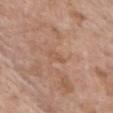{"biopsy_status": "not biopsied; imaged during a skin examination", "lighting": "white-light", "patient": {"sex": "female", "age_approx": 75}, "image": {"source": "total-body photography crop", "field_of_view_mm": 15}, "automated_metrics": {"area_mm2_approx": 2.0, "eccentricity": 0.95, "shape_asymmetry": 0.4, "vs_skin_darker_L": 6.0, "vs_skin_contrast_norm": 4.5, "nevus_likeness_0_100": 0, "lesion_detection_confidence_0_100": 100}, "site": "chest"}